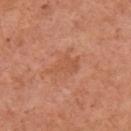The lesion was tiled from a total-body skin photograph and was not biopsied. The lesion is on the left arm. This image is a 15 mm lesion crop taken from a total-body photograph. The subject is a female aged 63 to 67.